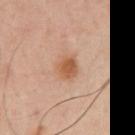Clinical impression: Imaged during a routine full-body skin examination; the lesion was not biopsied and no histopathology is available. Background: The subject is a male roughly 40 years of age. The total-body-photography lesion software estimated a border-irregularity index near 2/10, internal color variation of about 2.5 on a 0–10 scale, and peripheral color asymmetry of about 1. The software also gave an automated nevus-likeness rating near 95 out of 100. Imaged with cross-polarized lighting. The lesion is on the right upper arm. About 3 mm across. This image is a 15 mm lesion crop taken from a total-body photograph.